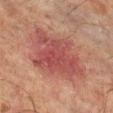workup=catalogued during a skin exam; not biopsied | automated metrics=a footprint of about 27 mm², a shape eccentricity near 0.6, and a symmetry-axis asymmetry near 0.35; a mean CIELAB color near L≈38 a*≈24 b*≈21 and a lesion–skin lightness drop of about 8; a nevus-likeness score of about 5/100 | anatomic site=the leg | illumination=cross-polarized illumination | patient=male, about 65 years old | diameter=~7 mm (longest diameter) | image=total-body-photography crop, ~15 mm field of view.